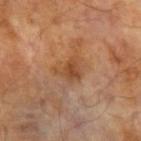  biopsy_status: not biopsied; imaged during a skin examination
  site: arm
  patient:
    sex: male
    age_approx: 70
  image:
    source: total-body photography crop
    field_of_view_mm: 15